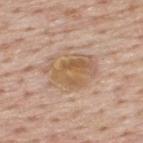biopsy status: catalogued during a skin exam; not biopsied
image source: 15 mm crop, total-body photography
patient: male, in their mid-70s
body site: the upper back
automated metrics: about 9 CIELAB-L* units darker than the surrounding skin; border irregularity of about 3 on a 0–10 scale and peripheral color asymmetry of about 2
lighting: white-light
size: about 6 mm